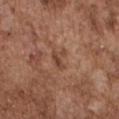A 15 mm crop from a total-body photograph taken for skin-cancer surveillance. A male patient in their mid-70s. Automated tile analysis of the lesion measured a lesion area of about 3.5 mm² and an eccentricity of roughly 0.85. The analysis additionally found an average lesion color of about L≈44 a*≈20 b*≈29 (CIELAB) and a normalized border contrast of about 6.5. The software also gave lesion-presence confidence of about 100/100. Imaged with white-light lighting. The lesion is located on the chest.